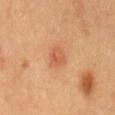Assessment: Captured during whole-body skin photography for melanoma surveillance; the lesion was not biopsied. Acquisition and patient details: The patient is a male approximately 60 years of age. From the abdomen. Cropped from a whole-body photographic skin survey; the tile spans about 15 mm.